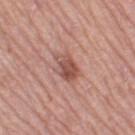patient:
  sex: female
  age_approx: 65
site: left thigh
image:
  source: total-body photography crop
  field_of_view_mm: 15
automated_metrics:
  area_mm2_approx: 5.5
  eccentricity: 0.75
  shape_asymmetry: 0.25
  cielab_L: 51
  cielab_a: 24
  cielab_b: 26
  vs_skin_darker_L: 11.0
  vs_skin_contrast_norm: 8.0
  border_irregularity_0_10: 2.5
  color_variation_0_10: 4.5
  peripheral_color_asymmetry: 1.5
  nevus_likeness_0_100: 60
  lesion_detection_confidence_0_100: 100